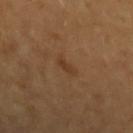No biopsy was performed on this lesion — it was imaged during a full skin examination and was not determined to be concerning. The tile uses cross-polarized illumination. Cropped from a whole-body photographic skin survey; the tile spans about 15 mm. The lesion is on the left forearm. The lesion's longest dimension is about 2.5 mm. Automated image analysis of the tile measured a footprint of about 2.5 mm², an outline eccentricity of about 0.9 (0 = round, 1 = elongated), and a shape-asymmetry score of about 0.4 (0 = symmetric). And it measured a mean CIELAB color near L≈38 a*≈19 b*≈33, a lesion–skin lightness drop of about 6, and a normalized lesion–skin contrast near 6. The software also gave a nevus-likeness score of about 10/100 and a lesion-detection confidence of about 100/100. The subject is a female aged 53–57.